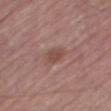workup=no biopsy performed (imaged during a skin exam) | body site=the right thigh | image=total-body-photography crop, ~15 mm field of view | automated metrics=a shape eccentricity near 0.75 and two-axis asymmetry of about 0.2; a border-irregularity index near 2/10 and internal color variation of about 1.5 on a 0–10 scale; a nevus-likeness score of about 5/100 and a lesion-detection confidence of about 100/100 | tile lighting=white-light | patient=male, about 75 years old | size=≈2.5 mm.